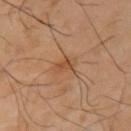  biopsy_status: not biopsied; imaged during a skin examination
  site: left upper arm
  image:
    source: total-body photography crop
    field_of_view_mm: 15
  lighting: cross-polarized
  patient:
    sex: male
    age_approx: 50
  lesion_size:
    long_diameter_mm_approx: 2.5